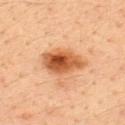Assessment: The lesion was photographed on a routine skin check and not biopsied; there is no pathology result. Acquisition and patient details: The recorded lesion diameter is about 5 mm. The total-body-photography lesion software estimated an average lesion color of about L≈52 a*≈25 b*≈38 (CIELAB), about 15 CIELAB-L* units darker than the surrounding skin, and a normalized border contrast of about 10.5. And it measured internal color variation of about 8 on a 0–10 scale. The software also gave an automated nevus-likeness rating near 100 out of 100 and a lesion-detection confidence of about 100/100. A region of skin cropped from a whole-body photographic capture, roughly 15 mm wide. A male subject, in their mid-30s. Located on the upper back.